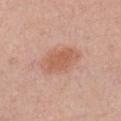workup: no biopsy performed (imaged during a skin exam) | location: the chest | tile lighting: white-light | patient: female, roughly 40 years of age | image-analysis metrics: an area of roughly 11 mm², an outline eccentricity of about 0.8 (0 = round, 1 = elongated), and a symmetry-axis asymmetry near 0.2 | acquisition: 15 mm crop, total-body photography | lesion size: ≈5 mm.